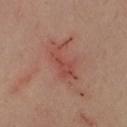Imaged during a routine full-body skin examination; the lesion was not biopsied and no histopathology is available. The lesion's longest dimension is about 5 mm. A roughly 15 mm field-of-view crop from a total-body skin photograph. A female patient in their 40s. The tile uses cross-polarized illumination. Located on the right forearm. Automated tile analysis of the lesion measured a footprint of about 8.5 mm², an eccentricity of roughly 0.85, and two-axis asymmetry of about 0.45.